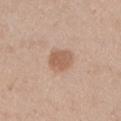Located on the left upper arm.
Measured at roughly 3 mm in maximum diameter.
The patient is a male in their mid-40s.
The total-body-photography lesion software estimated a border-irregularity rating of about 1/10, a within-lesion color-variation index near 2/10, and a peripheral color-asymmetry measure near 0.5.
Cropped from a whole-body photographic skin survey; the tile spans about 15 mm.